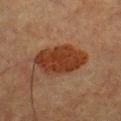Captured during whole-body skin photography for melanoma surveillance; the lesion was not biopsied.
Located on the chest.
A lesion tile, about 15 mm wide, cut from a 3D total-body photograph.
A male subject, aged approximately 50.
The tile uses cross-polarized illumination.
Automated image analysis of the tile measured an area of roughly 17 mm², a shape eccentricity near 0.85, and two-axis asymmetry of about 0.15. It also reported border irregularity of about 2 on a 0–10 scale, internal color variation of about 2.5 on a 0–10 scale, and a peripheral color-asymmetry measure near 1.